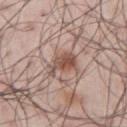follow-up: catalogued during a skin exam; not biopsied | subject: male, aged approximately 45 | TBP lesion metrics: an area of roughly 7 mm², an eccentricity of roughly 0.7, and a symmetry-axis asymmetry near 0.35; an average lesion color of about L≈52 a*≈19 b*≈25 (CIELAB) and about 12 CIELAB-L* units darker than the surrounding skin; a border-irregularity rating of about 4/10 and peripheral color asymmetry of about 1.5; a nevus-likeness score of about 90/100 and a detector confidence of about 100 out of 100 that the crop contains a lesion | image: total-body-photography crop, ~15 mm field of view | body site: the right upper arm.